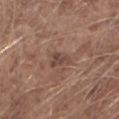- biopsy status — total-body-photography surveillance lesion; no biopsy
- site — the right forearm
- imaging modality — ~15 mm crop, total-body skin-cancer survey
- patient — male, aged 58 to 62
- tile lighting — white-light
- diameter — ~2.5 mm (longest diameter)